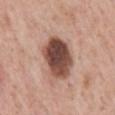{
  "biopsy_status": "not biopsied; imaged during a skin examination"
}